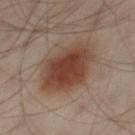biopsy status = total-body-photography surveillance lesion; no biopsy
lesion size = ~7 mm (longest diameter)
body site = the left leg
image source = 15 mm crop, total-body photography
patient = male, about 50 years old